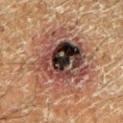Imaged with cross-polarized lighting. The recorded lesion diameter is about 9 mm. On the left lower leg. A close-up tile cropped from a whole-body skin photograph, about 15 mm across. The subject is a male about 60 years old.